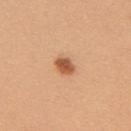Measured at roughly 2.5 mm in maximum diameter.
The lesion is on the back.
The patient is a female about 25 years old.
An algorithmic analysis of the crop reported a classifier nevus-likeness of about 100/100 and a lesion-detection confidence of about 100/100.
A lesion tile, about 15 mm wide, cut from a 3D total-body photograph.